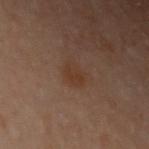The lesion was tiled from a total-body skin photograph and was not biopsied.
A region of skin cropped from a whole-body photographic capture, roughly 15 mm wide.
A female patient, approximately 60 years of age.
Captured under cross-polarized illumination.
From the right arm.